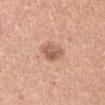Notes:
• notes — imaged on a skin check; not biopsied
• size — about 3 mm
• acquisition — 15 mm crop, total-body photography
• body site — the left upper arm
• automated metrics — a footprint of about 6.5 mm²; lesion-presence confidence of about 100/100
• subject — male, in their mid-20s
• tile lighting — white-light